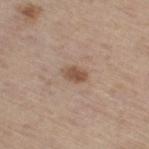Q: What lighting was used for the tile?
A: white-light illumination
Q: Patient demographics?
A: male, about 70 years old
Q: What is the anatomic site?
A: the right thigh
Q: What kind of image is this?
A: ~15 mm tile from a whole-body skin photo
Q: How large is the lesion?
A: ≈3 mm